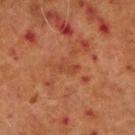Part of a total-body skin-imaging series; this lesion was reviewed on a skin check and was not flagged for biopsy.
The lesion is located on the right upper arm.
Measured at roughly 3 mm in maximum diameter.
The lesion-visualizer software estimated a mean CIELAB color near L≈37 a*≈24 b*≈30, about 5 CIELAB-L* units darker than the surrounding skin, and a normalized lesion–skin contrast near 4.5. The analysis additionally found a lesion-detection confidence of about 100/100.
Imaged with cross-polarized lighting.
A 15 mm close-up tile from a total-body photography series done for melanoma screening.
A male patient, approximately 70 years of age.